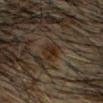The lesion was photographed on a routine skin check and not biopsied; there is no pathology result.
Longest diameter approximately 3 mm.
The tile uses cross-polarized illumination.
A 15 mm crop from a total-body photograph taken for skin-cancer surveillance.
The lesion-visualizer software estimated an area of roughly 3.5 mm², a shape eccentricity near 0.8, and two-axis asymmetry of about 0.25.
The patient is a male roughly 60 years of age.
From the head or neck.